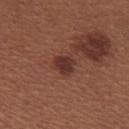Q: Is there a histopathology result?
A: catalogued during a skin exam; not biopsied
Q: Automated lesion metrics?
A: an area of roughly 5.5 mm², a shape eccentricity near 0.45, and two-axis asymmetry of about 0.3; a lesion color around L≈34 a*≈22 b*≈23 in CIELAB, a lesion–skin lightness drop of about 9, and a lesion-to-skin contrast of about 8.5 (normalized; higher = more distinct); a border-irregularity index near 2.5/10, internal color variation of about 2.5 on a 0–10 scale, and peripheral color asymmetry of about 1; an automated nevus-likeness rating near 70 out of 100
Q: Where on the body is the lesion?
A: the upper back
Q: Patient demographics?
A: female, aged 23 to 27
Q: What lighting was used for the tile?
A: white-light
Q: How was this image acquired?
A: total-body-photography crop, ~15 mm field of view
Q: Lesion size?
A: about 3 mm